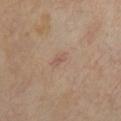The lesion was photographed on a routine skin check and not biopsied; there is no pathology result. The patient is a female aged approximately 50. The lesion is on the chest. A region of skin cropped from a whole-body photographic capture, roughly 15 mm wide. Captured under cross-polarized illumination.